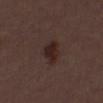Impression: Captured during whole-body skin photography for melanoma surveillance; the lesion was not biopsied. Background: A lesion tile, about 15 mm wide, cut from a 3D total-body photograph. The lesion is on the chest. The patient is a male aged 28 to 32.